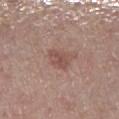{"biopsy_status": "not biopsied; imaged during a skin examination", "lighting": "white-light", "patient": {"sex": "female", "age_approx": 65}, "lesion_size": {"long_diameter_mm_approx": 3.0}, "site": "leg", "image": {"source": "total-body photography crop", "field_of_view_mm": 15}}